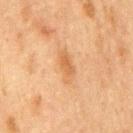Findings:
– workup · no biopsy performed (imaged during a skin exam)
– patient · male, aged around 75
– acquisition · ~15 mm tile from a whole-body skin photo
– location · the chest
– TBP lesion metrics · a border-irregularity index near 2.5/10, a color-variation rating of about 2.5/10, and peripheral color asymmetry of about 1